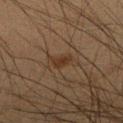follow-up: imaged on a skin check; not biopsied
lesion diameter: ~3 mm (longest diameter)
patient: male, roughly 35 years of age
acquisition: ~15 mm tile from a whole-body skin photo
body site: the right lower leg
tile lighting: cross-polarized illumination